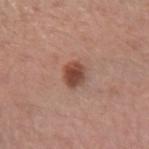No biopsy was performed on this lesion — it was imaged during a full skin examination and was not determined to be concerning.
Cropped from a whole-body photographic skin survey; the tile spans about 15 mm.
A female patient aged 48–52.
From the right forearm.
The recorded lesion diameter is about 2.5 mm.